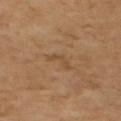{
  "biopsy_status": "not biopsied; imaged during a skin examination",
  "lighting": "cross-polarized",
  "image": {
    "source": "total-body photography crop",
    "field_of_view_mm": 15
  },
  "lesion_size": {
    "long_diameter_mm_approx": 3.5
  },
  "patient": {
    "sex": "male",
    "age_approx": 65
  },
  "automated_metrics": {
    "cielab_L": 46,
    "cielab_a": 18,
    "cielab_b": 33,
    "vs_skin_darker_L": 6.0,
    "vs_skin_contrast_norm": 5.0,
    "nevus_likeness_0_100": 0
  }
}